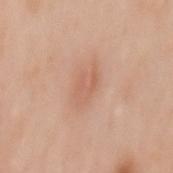• follow-up: total-body-photography surveillance lesion; no biopsy
• acquisition: 15 mm crop, total-body photography
• location: the mid back
• image-analysis metrics: about 7 CIELAB-L* units darker than the surrounding skin and a normalized border contrast of about 5; a border-irregularity index near 3/10, a color-variation rating of about 1.5/10, and peripheral color asymmetry of about 0.5
• lesion size: ~4 mm (longest diameter)
• tile lighting: white-light illumination
• patient: female, approximately 50 years of age